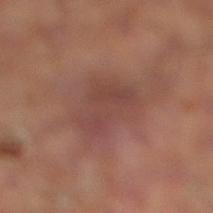No biopsy was performed on this lesion — it was imaged during a full skin examination and was not determined to be concerning. The lesion is on the right lower leg. A roughly 15 mm field-of-view crop from a total-body skin photograph. The lesion-visualizer software estimated a lesion area of about 15 mm² and a shape-asymmetry score of about 0.35 (0 = symmetric). The analysis additionally found a border-irregularity index near 4/10 and a color-variation rating of about 3/10. The software also gave a detector confidence of about 95 out of 100 that the crop contains a lesion. The subject is a male in their mid- to late 60s.